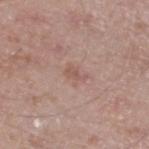Assessment:
Imaged during a routine full-body skin examination; the lesion was not biopsied and no histopathology is available.
Background:
This is a white-light tile. The lesion's longest dimension is about 2.5 mm. The lesion is on the left lower leg. The patient is a male approximately 50 years of age. Cropped from a total-body skin-imaging series; the visible field is about 15 mm. Automated image analysis of the tile measured a lesion color around L≈55 a*≈20 b*≈24 in CIELAB, roughly 7 lightness units darker than nearby skin, and a normalized border contrast of about 5. It also reported a lesion-detection confidence of about 100/100.